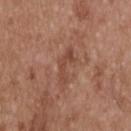Impression:
Captured during whole-body skin photography for melanoma surveillance; the lesion was not biopsied.
Context:
A male subject, about 55 years old. A lesion tile, about 15 mm wide, cut from a 3D total-body photograph. Imaged with white-light lighting. About 4 mm across. The lesion is located on the back.